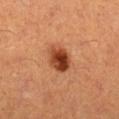Clinical impression: Part of a total-body skin-imaging series; this lesion was reviewed on a skin check and was not flagged for biopsy. Context: On the left leg. A female patient in their 30s. A 15 mm close-up tile from a total-body photography series done for melanoma screening. Longest diameter approximately 4 mm.